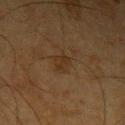Q: Was this lesion biopsied?
A: imaged on a skin check; not biopsied
Q: What did automated image analysis measure?
A: an eccentricity of roughly 0.7 and two-axis asymmetry of about 0.35
Q: What is the anatomic site?
A: the left upper arm
Q: What are the patient's age and sex?
A: male, approximately 65 years of age
Q: Illumination type?
A: cross-polarized illumination
Q: How was this image acquired?
A: 15 mm crop, total-body photography